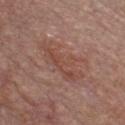Captured during whole-body skin photography for melanoma surveillance; the lesion was not biopsied. Measured at roughly 7 mm in maximum diameter. The patient is a male approximately 55 years of age. This is a white-light tile. The lesion is located on the chest. This image is a 15 mm lesion crop taken from a total-body photograph.